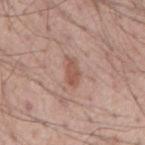The lesion was photographed on a routine skin check and not biopsied; there is no pathology result. Captured under white-light illumination. The subject is a male aged 53–57. A lesion tile, about 15 mm wide, cut from a 3D total-body photograph. The lesion is on the mid back. Longest diameter approximately 3.5 mm. An algorithmic analysis of the crop reported a symmetry-axis asymmetry near 0.25. The analysis additionally found a border-irregularity index near 2.5/10, internal color variation of about 1.5 on a 0–10 scale, and a peripheral color-asymmetry measure near 0.5. It also reported an automated nevus-likeness rating near 0 out of 100 and a detector confidence of about 100 out of 100 that the crop contains a lesion.